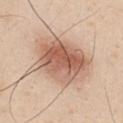Clinical impression: No biopsy was performed on this lesion — it was imaged during a full skin examination and was not determined to be concerning. Acquisition and patient details: About 8 mm across. A region of skin cropped from a whole-body photographic capture, roughly 15 mm wide. This is a white-light tile. Automated tile analysis of the lesion measured an automated nevus-likeness rating near 95 out of 100 and a lesion-detection confidence of about 100/100. From the upper back. A male patient, aged 23–27.